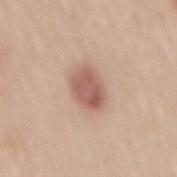follow-up: catalogued during a skin exam; not biopsied | subject: female, approximately 60 years of age | site: the back | imaging modality: ~15 mm crop, total-body skin-cancer survey | size: ~4 mm (longest diameter).